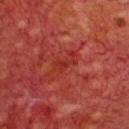Case summary:
• biopsy status — no biopsy performed (imaged during a skin exam)
• tile lighting — cross-polarized
• subject — male, aged 68 to 72
• size — ≈2.5 mm
• location — the chest
• image — ~15 mm tile from a whole-body skin photo